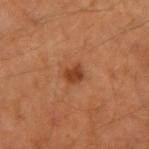Recorded during total-body skin imaging; not selected for excision or biopsy. From the right upper arm. The total-body-photography lesion software estimated an average lesion color of about L≈39 a*≈25 b*≈34 (CIELAB), about 10 CIELAB-L* units darker than the surrounding skin, and a normalized lesion–skin contrast near 8.5. The analysis additionally found a border-irregularity index near 2.5/10, internal color variation of about 2 on a 0–10 scale, and a peripheral color-asymmetry measure near 0.5. This is a cross-polarized tile. The lesion's longest dimension is about 2.5 mm. A close-up tile cropped from a whole-body skin photograph, about 15 mm across. A male subject aged 58 to 62.